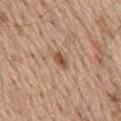Imaged during a routine full-body skin examination; the lesion was not biopsied and no histopathology is available.
A roughly 15 mm field-of-view crop from a total-body skin photograph.
The total-body-photography lesion software estimated an area of roughly 3 mm², an eccentricity of roughly 0.85, and a symmetry-axis asymmetry near 0.25.
Captured under white-light illumination.
A male patient aged 68 to 72.
On the back.